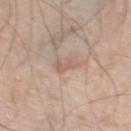Imaged during a routine full-body skin examination; the lesion was not biopsied and no histopathology is available.
The subject is a male aged 68–72.
This image is a 15 mm lesion crop taken from a total-body photograph.
From the left thigh.
This is a white-light tile.